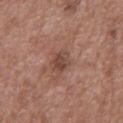{"biopsy_status": "not biopsied; imaged during a skin examination", "patient": {"sex": "male", "age_approx": 50}, "site": "back", "image": {"source": "total-body photography crop", "field_of_view_mm": 15}, "lighting": "white-light", "automated_metrics": {"area_mm2_approx": 4.0, "shape_asymmetry": 0.25}, "lesion_size": {"long_diameter_mm_approx": 2.5}}